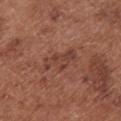notes: catalogued during a skin exam; not biopsied | lesion diameter: ~4.5 mm (longest diameter) | location: the chest | image-analysis metrics: a shape-asymmetry score of about 0.4 (0 = symmetric); roughly 7 lightness units darker than nearby skin and a lesion-to-skin contrast of about 6 (normalized; higher = more distinct); a border-irregularity rating of about 5.5/10, a within-lesion color-variation index near 2/10, and a peripheral color-asymmetry measure near 0.5; a classifier nevus-likeness of about 0/100 and a detector confidence of about 100 out of 100 that the crop contains a lesion | illumination: white-light | subject: female, aged approximately 75 | image source: 15 mm crop, total-body photography.